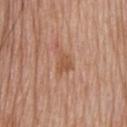The lesion was tiled from a total-body skin photograph and was not biopsied. The total-body-photography lesion software estimated border irregularity of about 4 on a 0–10 scale, a within-lesion color-variation index near 2/10, and a peripheral color-asymmetry measure near 0.5. On the upper back. Imaged with white-light lighting. A region of skin cropped from a whole-body photographic capture, roughly 15 mm wide. The subject is a male approximately 80 years of age. About 3 mm across.